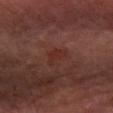<lesion>
  <biopsy_status>not biopsied; imaged during a skin examination</biopsy_status>
  <patient>
    <sex>male</sex>
    <age_approx>60</age_approx>
  </patient>
  <site>right forearm</site>
  <image>
    <source>total-body photography crop</source>
    <field_of_view_mm>15</field_of_view_mm>
  </image>
  <lighting>cross-polarized</lighting>
  <lesion_size>
    <long_diameter_mm_approx>3.0</long_diameter_mm_approx>
  </lesion_size>
</lesion>